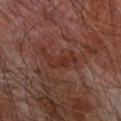Notes:
– workup — catalogued during a skin exam; not biopsied
– anatomic site — the right forearm
– lighting — cross-polarized illumination
– subject — male, about 65 years old
– imaging modality — ~15 mm tile from a whole-body skin photo
– automated metrics — an outline eccentricity of about 0.85 (0 = round, 1 = elongated)